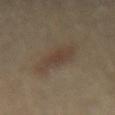Q: Was this lesion biopsied?
A: total-body-photography surveillance lesion; no biopsy
Q: What are the patient's age and sex?
A: female, roughly 60 years of age
Q: Lesion size?
A: about 5.5 mm
Q: What is the anatomic site?
A: the lower back
Q: What is the imaging modality?
A: ~15 mm tile from a whole-body skin photo
Q: What lighting was used for the tile?
A: cross-polarized illumination
Q: Automated lesion metrics?
A: a footprint of about 11 mm² and an eccentricity of roughly 0.85; a lesion color around L≈36 a*≈11 b*≈22 in CIELAB, roughly 6 lightness units darker than nearby skin, and a normalized lesion–skin contrast near 5.5; a border-irregularity index near 3/10 and a within-lesion color-variation index near 2.5/10